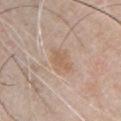  patient:
    sex: male
    age_approx: 50
  site: front of the torso
  image:
    source: total-body photography crop
    field_of_view_mm: 15
  automated_metrics:
    area_mm2_approx: 6.5
    eccentricity: 0.75
    shape_asymmetry: 0.3
    border_irregularity_0_10: 3.0
    color_variation_0_10: 2.0
  lighting: white-light